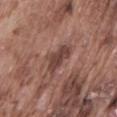Q: Was a biopsy performed?
A: imaged on a skin check; not biopsied
Q: Who is the patient?
A: male, about 75 years old
Q: What is the imaging modality?
A: ~15 mm crop, total-body skin-cancer survey
Q: What is the anatomic site?
A: the lower back
Q: What lighting was used for the tile?
A: white-light
Q: Lesion size?
A: about 4 mm
Q: Automated lesion metrics?
A: an outline eccentricity of about 0.85 (0 = round, 1 = elongated) and two-axis asymmetry of about 0.35; an average lesion color of about L≈42 a*≈20 b*≈23 (CIELAB) and a lesion–skin lightness drop of about 11